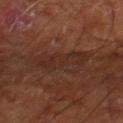Assessment:
Recorded during total-body skin imaging; not selected for excision or biopsy.
Context:
Approximately 6.5 mm at its widest. The lesion is located on the right lower leg. The subject is in their mid- to late 60s. Imaged with cross-polarized lighting. A lesion tile, about 15 mm wide, cut from a 3D total-body photograph.